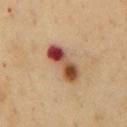Notes:
* follow-up · total-body-photography surveillance lesion; no biopsy
* imaging modality · 15 mm crop, total-body photography
* subject · male, in their 50s
* lighting · cross-polarized
* body site · the chest